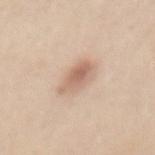Q: Was this lesion biopsied?
A: no biopsy performed (imaged during a skin exam)
Q: How was this image acquired?
A: ~15 mm crop, total-body skin-cancer survey
Q: Who is the patient?
A: female, aged around 45
Q: What lighting was used for the tile?
A: white-light
Q: How large is the lesion?
A: ≈4 mm
Q: Lesion location?
A: the mid back
Q: Automated lesion metrics?
A: an area of roughly 7 mm², an eccentricity of roughly 0.85, and a symmetry-axis asymmetry near 0.3; lesion-presence confidence of about 100/100